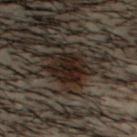Impression:
This lesion was catalogued during total-body skin photography and was not selected for biopsy.
Image and clinical context:
Cropped from a whole-body photographic skin survey; the tile spans about 15 mm. The lesion is on the head or neck. The tile uses cross-polarized illumination. A male subject in their 30s. The lesion-visualizer software estimated a lesion color around L≈15 a*≈9 b*≈14 in CIELAB, a lesion–skin lightness drop of about 9, and a normalized border contrast of about 12. It also reported a classifier nevus-likeness of about 40/100 and a detector confidence of about 75 out of 100 that the crop contains a lesion. Longest diameter approximately 5 mm.